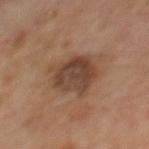– site — the mid back
– patient — male, approximately 65 years of age
– lighting — cross-polarized
– automated lesion analysis — a lesion area of about 15 mm², a shape eccentricity near 0.65, and two-axis asymmetry of about 0.25; a mean CIELAB color near L≈41 a*≈18 b*≈27, a lesion–skin lightness drop of about 10, and a normalized lesion–skin contrast near 8.5; a lesion-detection confidence of about 100/100
– acquisition — total-body-photography crop, ~15 mm field of view